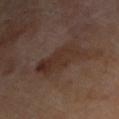biopsy status — imaged on a skin check; not biopsied | patient — male, aged 68 to 72 | automated metrics — an average lesion color of about L≈29 a*≈15 b*≈22 (CIELAB) and a normalized border contrast of about 6.5 | size — ≈6.5 mm | image — 15 mm crop, total-body photography | location — the right upper arm | illumination — cross-polarized illumination.